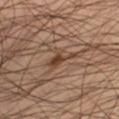Q: Automated lesion metrics?
A: a mean CIELAB color near L≈41 a*≈18 b*≈28, a lesion–skin lightness drop of about 9, and a lesion-to-skin contrast of about 7.5 (normalized; higher = more distinct)
Q: How large is the lesion?
A: ≈3.5 mm
Q: What is the anatomic site?
A: the right lower leg
Q: What are the patient's age and sex?
A: male, in their 40s
Q: How was the tile lit?
A: cross-polarized
Q: What is the imaging modality?
A: total-body-photography crop, ~15 mm field of view